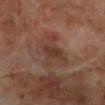Recorded during total-body skin imaging; not selected for excision or biopsy. A 15 mm close-up tile from a total-body photography series done for melanoma screening. The tile uses cross-polarized illumination. The subject is a male approximately 70 years of age. An algorithmic analysis of the crop reported a footprint of about 12 mm² and a shape-asymmetry score of about 0.45 (0 = symmetric). The software also gave a border-irregularity rating of about 6/10, internal color variation of about 3.5 on a 0–10 scale, and radial color variation of about 1. The lesion is on the leg.